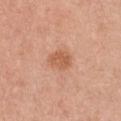Clinical impression: Imaged during a routine full-body skin examination; the lesion was not biopsied and no histopathology is available. Clinical summary: A female patient in their mid- to late 40s. The tile uses white-light illumination. Longest diameter approximately 3 mm. This image is a 15 mm lesion crop taken from a total-body photograph. From the chest. Automated image analysis of the tile measured border irregularity of about 2 on a 0–10 scale, a within-lesion color-variation index near 2/10, and radial color variation of about 0.5. It also reported an automated nevus-likeness rating near 20 out of 100.